A patient aged approximately 55 · located on the left upper arm · a roughly 15 mm field-of-view crop from a total-body skin photograph · captured under cross-polarized illumination · longest diameter approximately 1.5 mm.
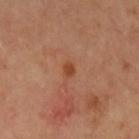pathology=a superficial basal cell carcinoma.